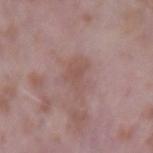Impression: Recorded during total-body skin imaging; not selected for excision or biopsy.